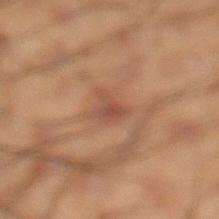This lesion was catalogued during total-body skin photography and was not selected for biopsy. A male patient about 50 years old. The lesion-visualizer software estimated a shape eccentricity near 0.7 and two-axis asymmetry of about 0.4. A 15 mm crop from a total-body photograph taken for skin-cancer surveillance. Measured at roughly 2.5 mm in maximum diameter. The lesion is on the left lower leg. The tile uses cross-polarized illumination.